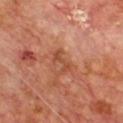biopsy status: catalogued during a skin exam; not biopsied
image: ~15 mm tile from a whole-body skin photo
anatomic site: the chest
patient: male, roughly 70 years of age
lesion size: ~3 mm (longest diameter)